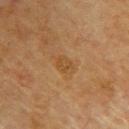biopsy status = catalogued during a skin exam; not biopsied.